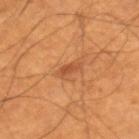The lesion was photographed on a routine skin check and not biopsied; there is no pathology result. About 3 mm across. A 15 mm close-up extracted from a 3D total-body photography capture. Located on the right lower leg. A male patient, aged around 55.